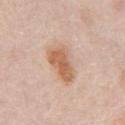This lesion was catalogued during total-body skin photography and was not selected for biopsy. A male subject aged around 75. A close-up tile cropped from a whole-body skin photograph, about 15 mm across. Approximately 5 mm at its widest. From the chest. This is a white-light tile.